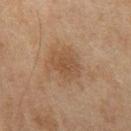- follow-up — catalogued during a skin exam; not biopsied
- automated metrics — a footprint of about 8 mm², an outline eccentricity of about 0.7 (0 = round, 1 = elongated), and two-axis asymmetry of about 0.15; a lesion color around L≈45 a*≈17 b*≈30 in CIELAB and a normalized border contrast of about 5.5; a border-irregularity rating of about 2/10, internal color variation of about 2 on a 0–10 scale, and peripheral color asymmetry of about 0.5; a classifier nevus-likeness of about 5/100 and a lesion-detection confidence of about 100/100
- subject — female, aged approximately 60
- site — the right thigh
- size — about 4 mm
- lighting — cross-polarized illumination
- acquisition — 15 mm crop, total-body photography